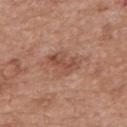| key | value |
|---|---|
| biopsy status | total-body-photography surveillance lesion; no biopsy |
| site | the mid back |
| TBP lesion metrics | a footprint of about 7.5 mm² and an outline eccentricity of about 0.8 (0 = round, 1 = elongated); a lesion–skin lightness drop of about 9 and a normalized border contrast of about 6.5; a border-irregularity rating of about 3.5/10 and a peripheral color-asymmetry measure near 1.5; a classifier nevus-likeness of about 0/100 and a lesion-detection confidence of about 100/100 |
| image source | ~15 mm tile from a whole-body skin photo |
| size | ≈4 mm |
| patient | male, aged 48–52 |